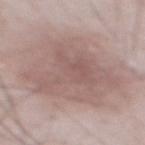Assessment: Recorded during total-body skin imaging; not selected for excision or biopsy. Background: On the abdomen. The tile uses white-light illumination. A 15 mm close-up extracted from a 3D total-body photography capture. A male subject, approximately 55 years of age.